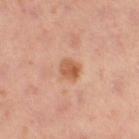Impression: No biopsy was performed on this lesion — it was imaged during a full skin examination and was not determined to be concerning. Background: The total-body-photography lesion software estimated a symmetry-axis asymmetry near 0.25. The analysis additionally found border irregularity of about 2 on a 0–10 scale, internal color variation of about 3.5 on a 0–10 scale, and radial color variation of about 1. A female patient aged 38 to 42. From the left thigh. Imaged with cross-polarized lighting. About 2.5 mm across. A 15 mm crop from a total-body photograph taken for skin-cancer surveillance.